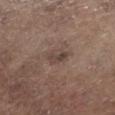{"biopsy_status": "not biopsied; imaged during a skin examination", "patient": {"sex": "male", "age_approx": 70}, "automated_metrics": {"area_mm2_approx": 3.5, "eccentricity": 0.85, "shape_asymmetry": 0.35, "cielab_L": 42, "cielab_a": 14, "cielab_b": 21, "vs_skin_darker_L": 8.0, "vs_skin_contrast_norm": 6.5, "nevus_likeness_0_100": 0, "lesion_detection_confidence_0_100": 100}, "image": {"source": "total-body photography crop", "field_of_view_mm": 15}, "site": "left lower leg", "lesion_size": {"long_diameter_mm_approx": 2.5}}